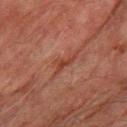Impression: This lesion was catalogued during total-body skin photography and was not selected for biopsy. Background: A region of skin cropped from a whole-body photographic capture, roughly 15 mm wide. Measured at roughly 2.5 mm in maximum diameter. On the right thigh. A male patient aged 73 to 77.